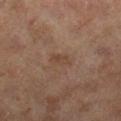A female subject about 60 years old.
This is a cross-polarized tile.
Automated image analysis of the tile measured a footprint of about 3.5 mm², an outline eccentricity of about 0.85 (0 = round, 1 = elongated), and a symmetry-axis asymmetry near 0.35.
A close-up tile cropped from a whole-body skin photograph, about 15 mm across.
From the right lower leg.
Measured at roughly 2.5 mm in maximum diameter.